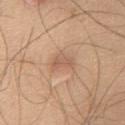Captured during whole-body skin photography for melanoma surveillance; the lesion was not biopsied. From the chest. Longest diameter approximately 3 mm. The tile uses white-light illumination. The total-body-photography lesion software estimated an eccentricity of roughly 0.75 and two-axis asymmetry of about 0.45. It also reported a lesion color around L≈59 a*≈20 b*≈31 in CIELAB, roughly 8 lightness units darker than nearby skin, and a normalized lesion–skin contrast near 5.5. The software also gave a peripheral color-asymmetry measure near 0.5. And it measured a classifier nevus-likeness of about 25/100 and lesion-presence confidence of about 100/100. The subject is a male aged approximately 45. A 15 mm close-up extracted from a 3D total-body photography capture.